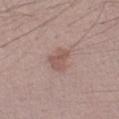biopsy status = no biopsy performed (imaged during a skin exam)
patient = male, aged around 25
lesion size = ≈3 mm
imaging modality = 15 mm crop, total-body photography
body site = the left forearm
lighting = white-light illumination